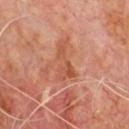This lesion was catalogued during total-body skin photography and was not selected for biopsy. Automated tile analysis of the lesion measured a lesion–skin lightness drop of about 8 and a normalized border contrast of about 6.5. The software also gave a nevus-likeness score of about 0/100 and a lesion-detection confidence of about 100/100. A roughly 15 mm field-of-view crop from a total-body skin photograph. This is a cross-polarized tile. Located on the chest. The patient is a male aged around 65. About 4.5 mm across.